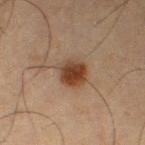Assessment:
The lesion was tiled from a total-body skin photograph and was not biopsied.
Clinical summary:
Cropped from a total-body skin-imaging series; the visible field is about 15 mm. From the left thigh. A male subject, aged 63 to 67. This is a cross-polarized tile. Measured at roughly 3.5 mm in maximum diameter.